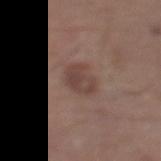notes — catalogued during a skin exam; not biopsied
diameter — ≈3.5 mm
automated lesion analysis — a footprint of about 8.5 mm², a shape eccentricity near 0.35, and a shape-asymmetry score of about 0.2 (0 = symmetric); a nevus-likeness score of about 10/100 and a lesion-detection confidence of about 100/100
anatomic site — the leg
imaging modality — 15 mm crop, total-body photography
subject — male, in their mid- to late 60s